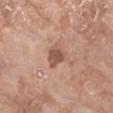follow-up: imaged on a skin check; not biopsied | anatomic site: the left forearm | illumination: white-light | patient: female, approximately 75 years of age | imaging modality: total-body-photography crop, ~15 mm field of view | size: about 2.5 mm.